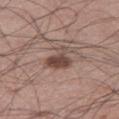notes: no biopsy performed (imaged during a skin exam) | illumination: white-light illumination | TBP lesion metrics: an average lesion color of about L≈45 a*≈18 b*≈22 (CIELAB), a lesion–skin lightness drop of about 12, and a lesion-to-skin contrast of about 9 (normalized; higher = more distinct); border irregularity of about 4.5 on a 0–10 scale, internal color variation of about 2.5 on a 0–10 scale, and radial color variation of about 0.5 | patient: male, roughly 55 years of age | imaging modality: ~15 mm crop, total-body skin-cancer survey | location: the right thigh.